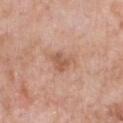image-analysis metrics=a within-lesion color-variation index near 2/10; acquisition=total-body-photography crop, ~15 mm field of view; anatomic site=the chest; patient=male, in their 60s; illumination=white-light; lesion size=≈3 mm.